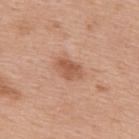Part of a total-body skin-imaging series; this lesion was reviewed on a skin check and was not flagged for biopsy.
The lesion is located on the upper back.
A 15 mm crop from a total-body photograph taken for skin-cancer surveillance.
The patient is a female aged 38 to 42.
Imaged with white-light lighting.
The total-body-photography lesion software estimated an average lesion color of about L≈56 a*≈24 b*≈33 (CIELAB) and a lesion–skin lightness drop of about 10. And it measured a nevus-likeness score of about 55/100 and a detector confidence of about 100 out of 100 that the crop contains a lesion.
About 3.5 mm across.